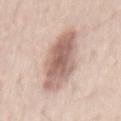body site: the mid back
image source: 15 mm crop, total-body photography
patient: male, approximately 40 years of age